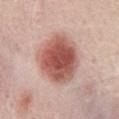* workup: total-body-photography surveillance lesion; no biopsy
* patient: male, aged 68 to 72
* anatomic site: the front of the torso
* automated lesion analysis: a lesion–skin lightness drop of about 17 and a normalized lesion–skin contrast near 11; a border-irregularity rating of about 1.5/10 and a peripheral color-asymmetry measure near 1.5; a lesion-detection confidence of about 100/100
* lesion diameter: about 6.5 mm
* illumination: white-light illumination
* image source: total-body-photography crop, ~15 mm field of view